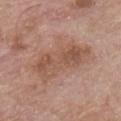follow-up: total-body-photography surveillance lesion; no biopsy | patient: male, aged 63–67 | tile lighting: white-light illumination | site: the chest | lesion size: ≈7 mm | acquisition: total-body-photography crop, ~15 mm field of view.